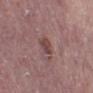Background:
On the left lower leg. Imaged with white-light lighting. Measured at roughly 2.5 mm in maximum diameter. A male subject aged 73–77. A 15 mm crop from a total-body photograph taken for skin-cancer surveillance.